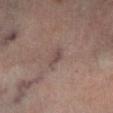* follow-up · catalogued during a skin exam; not biopsied